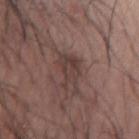Q: Was a biopsy performed?
A: no biopsy performed (imaged during a skin exam)
Q: Who is the patient?
A: male, aged approximately 65
Q: What is the anatomic site?
A: the right forearm
Q: How large is the lesion?
A: ~3.5 mm (longest diameter)
Q: Automated lesion metrics?
A: a mean CIELAB color near L≈39 a*≈16 b*≈19, a lesion–skin lightness drop of about 7, and a lesion-to-skin contrast of about 6.5 (normalized; higher = more distinct); a classifier nevus-likeness of about 0/100 and a lesion-detection confidence of about 95/100
Q: How was this image acquired?
A: ~15 mm crop, total-body skin-cancer survey
Q: Illumination type?
A: white-light